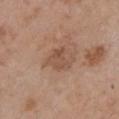{"biopsy_status": "not biopsied; imaged during a skin examination", "patient": {"sex": "female", "age_approx": 65}, "site": "chest", "image": {"source": "total-body photography crop", "field_of_view_mm": 15}}